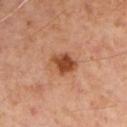follow-up = catalogued during a skin exam; not biopsied | lighting = cross-polarized | imaging modality = 15 mm crop, total-body photography | subject = male, in their mid- to late 50s | TBP lesion metrics = a mean CIELAB color near L≈48 a*≈26 b*≈36 and roughly 14 lightness units darker than nearby skin; a nevus-likeness score of about 90/100 | location = the left upper arm | diameter = about 3.5 mm.